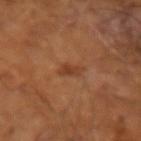notes = total-body-photography surveillance lesion; no biopsy
automated metrics = a mean CIELAB color near L≈39 a*≈23 b*≈33, roughly 7 lightness units darker than nearby skin, and a normalized lesion–skin contrast near 6.5; border irregularity of about 3 on a 0–10 scale and a peripheral color-asymmetry measure near 0.5; an automated nevus-likeness rating near 45 out of 100
patient = male, roughly 65 years of age
tile lighting = cross-polarized illumination
acquisition = ~15 mm crop, total-body skin-cancer survey